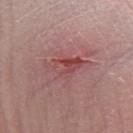Findings:
* biopsy status · imaged on a skin check; not biopsied
* patient · female, aged approximately 40
* anatomic site · the right forearm
* diameter · about 5.5 mm
* tile lighting · white-light
* image source · ~15 mm crop, total-body skin-cancer survey